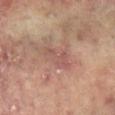Findings:
– notes — total-body-photography surveillance lesion; no biopsy
– location — the right arm
– patient — female, aged 78 to 82
– acquisition — ~15 mm tile from a whole-body skin photo